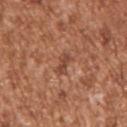| field | value |
|---|---|
| notes | imaged on a skin check; not biopsied |
| subject | male, aged 43 to 47 |
| automated lesion analysis | a footprint of about 3.5 mm² and a shape-asymmetry score of about 0.35 (0 = symmetric); a mean CIELAB color near L≈46 a*≈24 b*≈30 and a lesion–skin lightness drop of about 9; a border-irregularity index near 3.5/10 and a peripheral color-asymmetry measure near 0.5 |
| site | the right upper arm |
| imaging modality | 15 mm crop, total-body photography |
| illumination | white-light |